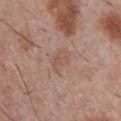Clinical impression: Captured during whole-body skin photography for melanoma surveillance; the lesion was not biopsied.